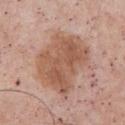Part of a total-body skin-imaging series; this lesion was reviewed on a skin check and was not flagged for biopsy. This image is a 15 mm lesion crop taken from a total-body photograph. From the chest. The subject is a male about 55 years old. Captured under white-light illumination. The recorded lesion diameter is about 7.5 mm.